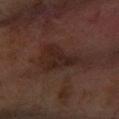  biopsy_status: not biopsied; imaged during a skin examination
  patient:
    sex: female
    age_approx: 60
  image:
    source: total-body photography crop
    field_of_view_mm: 15
  lesion_size:
    long_diameter_mm_approx: 6.5
  lighting: cross-polarized
  site: right forearm